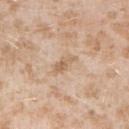The lesion was photographed on a routine skin check and not biopsied; there is no pathology result. The recorded lesion diameter is about 3 mm. Cropped from a whole-body photographic skin survey; the tile spans about 15 mm. The tile uses white-light illumination. Automated image analysis of the tile measured a footprint of about 3.5 mm², an outline eccentricity of about 0.9 (0 = round, 1 = elongated), and two-axis asymmetry of about 0.4. And it measured a mean CIELAB color near L≈63 a*≈17 b*≈33 and roughly 9 lightness units darker than nearby skin. And it measured a border-irregularity index near 4.5/10 and internal color variation of about 1 on a 0–10 scale. The patient is a female aged approximately 25. Located on the arm.